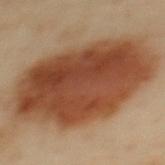follow-up: no biopsy performed (imaged during a skin exam)
lighting: cross-polarized
anatomic site: the upper back
TBP lesion metrics: an eccentricity of roughly 0.8 and a symmetry-axis asymmetry near 0.1; a mean CIELAB color near L≈39 a*≈20 b*≈29 and a lesion-to-skin contrast of about 11.5 (normalized; higher = more distinct); a border-irregularity index near 1.5/10, a color-variation rating of about 6/10, and peripheral color asymmetry of about 2; an automated nevus-likeness rating near 100 out of 100 and lesion-presence confidence of about 100/100
subject: female, in their 60s
image: 15 mm crop, total-body photography